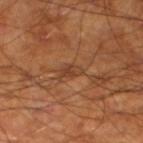Imaged during a routine full-body skin examination; the lesion was not biopsied and no histopathology is available. Approximately 2.5 mm at its widest. From the leg. The total-body-photography lesion software estimated an area of roughly 3 mm² and a shape eccentricity near 0.85. It also reported a mean CIELAB color near L≈40 a*≈22 b*≈32, about 7 CIELAB-L* units darker than the surrounding skin, and a lesion-to-skin contrast of about 6 (normalized; higher = more distinct). And it measured a border-irregularity index near 2.5/10, a color-variation rating of about 1/10, and a peripheral color-asymmetry measure near 0.5. The analysis additionally found an automated nevus-likeness rating near 0 out of 100 and a lesion-detection confidence of about 65/100. The patient is a male approximately 60 years of age. Imaged with cross-polarized lighting. A 15 mm close-up tile from a total-body photography series done for melanoma screening.